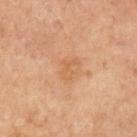Assessment: This lesion was catalogued during total-body skin photography and was not selected for biopsy. Clinical summary: Cropped from a total-body skin-imaging series; the visible field is about 15 mm. The lesion is located on the right upper arm. An algorithmic analysis of the crop reported a footprint of about 3 mm², a shape eccentricity near 0.75, and a shape-asymmetry score of about 0.4 (0 = symmetric). The analysis additionally found a border-irregularity rating of about 4.5/10, a color-variation rating of about 0/10, and radial color variation of about 0. The software also gave an automated nevus-likeness rating near 0 out of 100. The recorded lesion diameter is about 2.5 mm. The tile uses cross-polarized illumination. The patient is a male aged around 70.